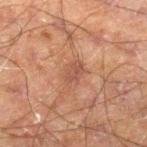This lesion was catalogued during total-body skin photography and was not selected for biopsy. This is a cross-polarized tile. From the left leg. A close-up tile cropped from a whole-body skin photograph, about 15 mm across. A male subject roughly 60 years of age.